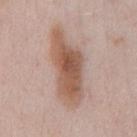The lesion was tiled from a total-body skin photograph and was not biopsied.
A 15 mm crop from a total-body photograph taken for skin-cancer surveillance.
Located on the chest.
The patient is a male in their mid-50s.